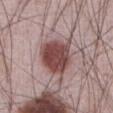• biopsy status · imaged on a skin check; not biopsied
• patient · male, about 65 years old
• lesion diameter · ≈4.5 mm
• anatomic site · the front of the torso
• imaging modality · total-body-photography crop, ~15 mm field of view
• tile lighting · white-light